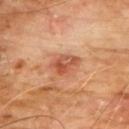Assessment: The lesion was tiled from a total-body skin photograph and was not biopsied. Image and clinical context: The lesion is located on the chest. The subject is a male aged around 60. The lesion's longest dimension is about 3.5 mm. A lesion tile, about 15 mm wide, cut from a 3D total-body photograph. An algorithmic analysis of the crop reported a classifier nevus-likeness of about 15/100.